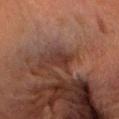| feature | finding |
|---|---|
| biopsy status | no biopsy performed (imaged during a skin exam) |
| illumination | cross-polarized |
| imaging modality | ~15 mm crop, total-body skin-cancer survey |
| lesion size | ~3 mm (longest diameter) |
| site | the head or neck |
| patient | male, about 45 years old |